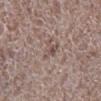biopsy_status: not biopsied; imaged during a skin examination
image:
  source: total-body photography crop
  field_of_view_mm: 15
site: right lower leg
patient:
  sex: male
  age_approx: 70